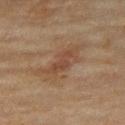Recorded during total-body skin imaging; not selected for excision or biopsy. A 15 mm crop from a total-body photograph taken for skin-cancer surveillance. Automated tile analysis of the lesion measured a lesion color around L≈42 a*≈17 b*≈28 in CIELAB and a normalized border contrast of about 6. The lesion is located on the right thigh. A female subject, aged approximately 60. Measured at roughly 6.5 mm in maximum diameter.